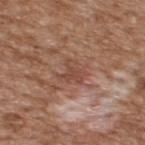biopsy_status: not biopsied; imaged during a skin examination
image:
  source: total-body photography crop
  field_of_view_mm: 15
lesion_size:
  long_diameter_mm_approx: 3.0
site: upper back
lighting: white-light
patient:
  sex: male
  age_approx: 65